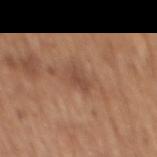Q: Patient demographics?
A: male, aged 63–67
Q: What is the imaging modality?
A: ~15 mm crop, total-body skin-cancer survey
Q: Automated lesion metrics?
A: a lesion area of about 4 mm², an eccentricity of roughly 0.8, and a shape-asymmetry score of about 0.25 (0 = symmetric); a classifier nevus-likeness of about 0/100 and a lesion-detection confidence of about 100/100
Q: Where on the body is the lesion?
A: the mid back
Q: How large is the lesion?
A: about 3 mm
Q: How was the tile lit?
A: white-light